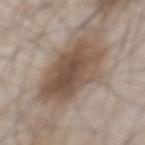Findings:
* follow-up — imaged on a skin check; not biopsied
* lesion diameter — ~8 mm (longest diameter)
* patient — male, aged around 55
* automated metrics — an average lesion color of about L≈50 a*≈14 b*≈25 (CIELAB), roughly 12 lightness units darker than nearby skin, and a normalized border contrast of about 8.5; a border-irregularity index near 2.5/10, a within-lesion color-variation index near 5/10, and radial color variation of about 1.5; an automated nevus-likeness rating near 50 out of 100
* image source — ~15 mm crop, total-body skin-cancer survey
* body site — the front of the torso